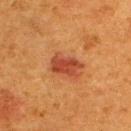Clinical impression:
Captured during whole-body skin photography for melanoma surveillance; the lesion was not biopsied.
Background:
Captured under cross-polarized illumination. A 15 mm close-up extracted from a 3D total-body photography capture. The lesion's longest dimension is about 4 mm. From the upper back. The subject is a female approximately 40 years of age. The total-body-photography lesion software estimated an area of roughly 8 mm² and a shape-asymmetry score of about 0.25 (0 = symmetric). The software also gave a border-irregularity index near 3/10, a within-lesion color-variation index near 3/10, and peripheral color asymmetry of about 1.